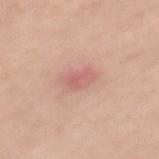{
  "biopsy_status": "not biopsied; imaged during a skin examination",
  "automated_metrics": {
    "vs_skin_contrast_norm": 5.5,
    "color_variation_0_10": 2.5,
    "peripheral_color_asymmetry": 1.0,
    "nevus_likeness_0_100": 0
  },
  "lesion_size": {
    "long_diameter_mm_approx": 3.0
  },
  "image": {
    "source": "total-body photography crop",
    "field_of_view_mm": 15
  },
  "patient": {
    "sex": "female",
    "age_approx": 50
  },
  "site": "back",
  "lighting": "white-light"
}